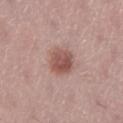notes: catalogued during a skin exam; not biopsied | lesion size: ~3.5 mm (longest diameter) | body site: the right lower leg | image source: 15 mm crop, total-body photography | image-analysis metrics: about 12 CIELAB-L* units darker than the surrounding skin and a normalized border contrast of about 8; border irregularity of about 1.5 on a 0–10 scale, a color-variation rating of about 4/10, and peripheral color asymmetry of about 1.5; lesion-presence confidence of about 100/100 | illumination: white-light illumination | subject: female, about 50 years old.